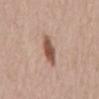notes: catalogued during a skin exam; not biopsied
image source: ~15 mm crop, total-body skin-cancer survey
TBP lesion metrics: a lesion area of about 7.5 mm², an eccentricity of roughly 0.85, and two-axis asymmetry of about 0.15; about 14 CIELAB-L* units darker than the surrounding skin; a border-irregularity index near 2/10, a within-lesion color-variation index near 3/10, and a peripheral color-asymmetry measure near 1
subject: female, approximately 65 years of age
lighting: white-light
lesion diameter: about 4 mm
site: the mid back